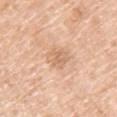{
  "biopsy_status": "not biopsied; imaged during a skin examination",
  "site": "left upper arm",
  "image": {
    "source": "total-body photography crop",
    "field_of_view_mm": 15
  },
  "patient": {
    "sex": "female",
    "age_approx": 45
  },
  "lighting": "white-light"
}